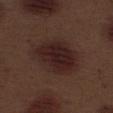Context: Automated tile analysis of the lesion measured an area of roughly 23 mm², an eccentricity of roughly 0.8, and two-axis asymmetry of about 0.2. The analysis additionally found an average lesion color of about L≈23 a*≈17 b*≈18 (CIELAB), a lesion–skin lightness drop of about 8, and a normalized border contrast of about 9.5. The software also gave border irregularity of about 2 on a 0–10 scale, a within-lesion color-variation index near 4/10, and peripheral color asymmetry of about 1. It also reported a nevus-likeness score of about 100/100 and a lesion-detection confidence of about 100/100. From the left thigh. About 7 mm across. A 15 mm crop from a total-body photograph taken for skin-cancer surveillance. A male subject, approximately 70 years of age.